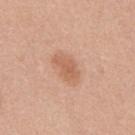This lesion was catalogued during total-body skin photography and was not selected for biopsy. A roughly 15 mm field-of-view crop from a total-body skin photograph. Imaged with white-light lighting. Approximately 4 mm at its widest. The total-body-photography lesion software estimated an area of roughly 6.5 mm², an eccentricity of roughly 0.9, and a shape-asymmetry score of about 0.25 (0 = symmetric). The software also gave border irregularity of about 3 on a 0–10 scale, a color-variation rating of about 2.5/10, and peripheral color asymmetry of about 1. A male patient aged 43 to 47. Located on the mid back.